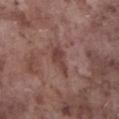Imaged during a routine full-body skin examination; the lesion was not biopsied and no histopathology is available. The total-body-photography lesion software estimated a mean CIELAB color near L≈41 a*≈20 b*≈22 and a normalized border contrast of about 7. The software also gave a classifier nevus-likeness of about 0/100 and a lesion-detection confidence of about 100/100. About 3.5 mm across. A region of skin cropped from a whole-body photographic capture, roughly 15 mm wide. A male patient approximately 75 years of age. On the left lower leg.